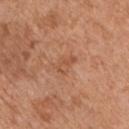Clinical summary:
A male patient, in their mid-50s. On the chest. A close-up tile cropped from a whole-body skin photograph, about 15 mm across. About 3 mm across. The tile uses white-light illumination.